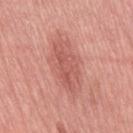Clinical impression:
Imaged during a routine full-body skin examination; the lesion was not biopsied and no histopathology is available.
Context:
A lesion tile, about 15 mm wide, cut from a 3D total-body photograph. This is a white-light tile. On the right thigh. The subject is a female aged around 40.